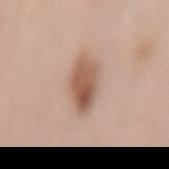Recorded during total-body skin imaging; not selected for excision or biopsy.
On the mid back.
Cropped from a whole-body photographic skin survey; the tile spans about 15 mm.
Approximately 5 mm at its widest.
A female subject aged approximately 50.